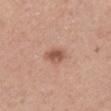The lesion was tiled from a total-body skin photograph and was not biopsied.